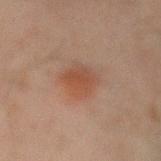follow-up: catalogued during a skin exam; not biopsied
acquisition: ~15 mm tile from a whole-body skin photo
body site: the back
tile lighting: cross-polarized
lesion size: about 4 mm
TBP lesion metrics: a lesion color around L≈40 a*≈17 b*≈25 in CIELAB, a lesion–skin lightness drop of about 6, and a lesion-to-skin contrast of about 6 (normalized; higher = more distinct); a border-irregularity index near 2.5/10 and a color-variation rating of about 3.5/10; an automated nevus-likeness rating near 100 out of 100
patient: male, in their mid-40s